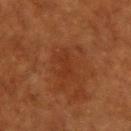• biopsy status: catalogued during a skin exam; not biopsied
• subject: female, roughly 80 years of age
• lesion diameter: about 3 mm
• image source: 15 mm crop, total-body photography
• tile lighting: cross-polarized illumination
• TBP lesion metrics: an area of roughly 4 mm² and a shape eccentricity near 0.85; a border-irregularity rating of about 4.5/10, internal color variation of about 0.5 on a 0–10 scale, and a peripheral color-asymmetry measure near 0; a nevus-likeness score of about 0/100 and a detector confidence of about 100 out of 100 that the crop contains a lesion
• anatomic site: the upper back